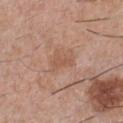{"biopsy_status": "not biopsied; imaged during a skin examination", "image": {"source": "total-body photography crop", "field_of_view_mm": 15}, "lighting": "white-light", "patient": {"sex": "male", "age_approx": 30}, "site": "front of the torso", "lesion_size": {"long_diameter_mm_approx": 2.5}}